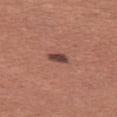{
  "biopsy_status": "not biopsied; imaged during a skin examination",
  "lighting": "white-light",
  "lesion_size": {
    "long_diameter_mm_approx": 2.5
  },
  "image": {
    "source": "total-body photography crop",
    "field_of_view_mm": 15
  },
  "automated_metrics": {
    "area_mm2_approx": 3.0,
    "eccentricity": 0.8,
    "shape_asymmetry": 0.25,
    "cielab_L": 43,
    "cielab_a": 22,
    "cielab_b": 23,
    "vs_skin_darker_L": 13.0,
    "vs_skin_contrast_norm": 10.5,
    "border_irregularity_0_10": 2.5,
    "color_variation_0_10": 1.5
  },
  "site": "left thigh",
  "patient": {
    "sex": "female",
    "age_approx": 50
  }
}